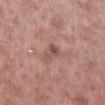Findings:
* notes — total-body-photography surveillance lesion; no biopsy
* patient — male, about 55 years old
* image — 15 mm crop, total-body photography
* anatomic site — the right lower leg